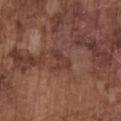Clinical summary: A lesion tile, about 15 mm wide, cut from a 3D total-body photograph. The patient is a male approximately 75 years of age. About 3 mm across. The total-body-photography lesion software estimated about 6 CIELAB-L* units darker than the surrounding skin and a lesion-to-skin contrast of about 5.5 (normalized; higher = more distinct). Located on the chest.